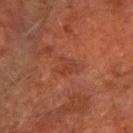| field | value |
|---|---|
| notes | imaged on a skin check; not biopsied |
| lighting | cross-polarized illumination |
| TBP lesion metrics | a footprint of about 5 mm² and an outline eccentricity of about 0.65 (0 = round, 1 = elongated); an average lesion color of about L≈33 a*≈24 b*≈28 (CIELAB), about 5 CIELAB-L* units darker than the surrounding skin, and a normalized border contrast of about 4.5; a color-variation rating of about 1.5/10; an automated nevus-likeness rating near 0 out of 100 and a lesion-detection confidence of about 100/100 |
| lesion diameter | ~3 mm (longest diameter) |
| site | the arm |
| patient | male, about 60 years old |
| image | 15 mm crop, total-body photography |